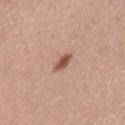<record>
  <lighting>white-light</lighting>
  <site>leg</site>
  <lesion_size>
    <long_diameter_mm_approx>2.5</long_diameter_mm_approx>
  </lesion_size>
  <image>
    <source>total-body photography crop</source>
    <field_of_view_mm>15</field_of_view_mm>
  </image>
  <automated_metrics>
    <cielab_L>53</cielab_L>
    <cielab_a>22</cielab_a>
    <cielab_b>28</cielab_b>
    <vs_skin_darker_L>14.0</vs_skin_darker_L>
    <vs_skin_contrast_norm>9.5</vs_skin_contrast_norm>
    <color_variation_0_10>1.5</color_variation_0_10>
    <nevus_likeness_0_100>90</nevus_likeness_0_100>
    <lesion_detection_confidence_0_100>100</lesion_detection_confidence_0_100>
  </automated_metrics>
  <patient>
    <sex>female</sex>
    <age_approx>45</age_approx>
  </patient>
</record>